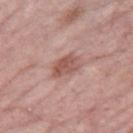<case>
<site>right thigh</site>
<lighting>white-light</lighting>
<patient>
  <sex>female</sex>
  <age_approx>70</age_approx>
</patient>
<image>
  <source>total-body photography crop</source>
  <field_of_view_mm>15</field_of_view_mm>
</image>
</case>